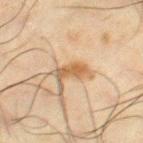No biopsy was performed on this lesion — it was imaged during a full skin examination and was not determined to be concerning.
The tile uses cross-polarized illumination.
A male subject about 65 years old.
From the right thigh.
Cropped from a total-body skin-imaging series; the visible field is about 15 mm.
About 3.5 mm across.